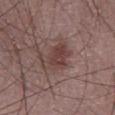Assessment:
No biopsy was performed on this lesion — it was imaged during a full skin examination and was not determined to be concerning.
Image and clinical context:
This image is a 15 mm lesion crop taken from a total-body photograph. The subject is a male aged approximately 60. From the abdomen.